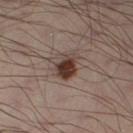• follow-up: total-body-photography surveillance lesion; no biopsy
• body site: the right thigh
• image source: ~15 mm tile from a whole-body skin photo
• lesion size: ≈4 mm
• patient: male, about 40 years old